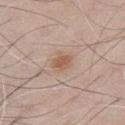patient:
  sex: male
  age_approx: 70
image:
  source: total-body photography crop
  field_of_view_mm: 15
site: chest